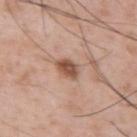size — about 3 mm | subject — male, aged 53–57 | location — the left upper arm | TBP lesion metrics — an average lesion color of about L≈52 a*≈21 b*≈29 (CIELAB), about 14 CIELAB-L* units darker than the surrounding skin, and a lesion-to-skin contrast of about 9.5 (normalized; higher = more distinct); a classifier nevus-likeness of about 90/100 and a lesion-detection confidence of about 100/100 | image source — total-body-photography crop, ~15 mm field of view.